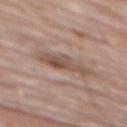notes = no biopsy performed (imaged during a skin exam) | patient = male, approximately 80 years of age | lesion diameter = ≈4.5 mm | image = ~15 mm tile from a whole-body skin photo | image-analysis metrics = a lesion color around L≈51 a*≈18 b*≈24 in CIELAB, a lesion–skin lightness drop of about 10, and a normalized border contrast of about 7; an automated nevus-likeness rating near 0 out of 100 and a lesion-detection confidence of about 100/100 | body site = the mid back.